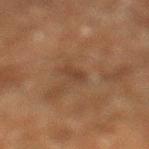Clinical impression:
No biopsy was performed on this lesion — it was imaged during a full skin examination and was not determined to be concerning.
Context:
Automated tile analysis of the lesion measured a footprint of about 2 mm², an eccentricity of roughly 0.9, and a symmetry-axis asymmetry near 0.3. The software also gave about 5 CIELAB-L* units darker than the surrounding skin. It also reported a border-irregularity index near 3.5/10. It also reported a nevus-likeness score of about 0/100 and a detector confidence of about 100 out of 100 that the crop contains a lesion. The subject is a male in their 60s. Longest diameter approximately 2.5 mm. This image is a 15 mm lesion crop taken from a total-body photograph. Located on the left lower leg. Captured under cross-polarized illumination.